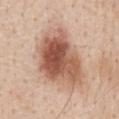Captured during whole-body skin photography for melanoma surveillance; the lesion was not biopsied. Located on the front of the torso. A male patient in their 60s. A close-up tile cropped from a whole-body skin photograph, about 15 mm across.